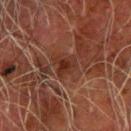<lesion>
  <biopsy_status>not biopsied; imaged during a skin examination</biopsy_status>
  <automated_metrics>
    <area_mm2_approx>5.5</area_mm2_approx>
    <eccentricity>0.35</eccentricity>
    <cielab_L>24</cielab_L>
    <cielab_a>19</cielab_a>
    <cielab_b>22</cielab_b>
    <vs_skin_darker_L>6.0</vs_skin_darker_L>
    <vs_skin_contrast_norm>7.0</vs_skin_contrast_norm>
    <border_irregularity_0_10>3.5</border_irregularity_0_10>
    <color_variation_0_10>2.5</color_variation_0_10>
    <peripheral_color_asymmetry>1.0</peripheral_color_asymmetry>
    <nevus_likeness_0_100>0</nevus_likeness_0_100>
  </automated_metrics>
  <site>arm</site>
  <patient>
    <sex>male</sex>
    <age_approx>80</age_approx>
  </patient>
  <lesion_size>
    <long_diameter_mm_approx>3.0</long_diameter_mm_approx>
  </lesion_size>
  <image>
    <source>total-body photography crop</source>
    <field_of_view_mm>15</field_of_view_mm>
  </image>
</lesion>